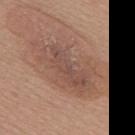Clinical impression: Recorded during total-body skin imaging; not selected for excision or biopsy. Background: A roughly 15 mm field-of-view crop from a total-body skin photograph. The total-body-photography lesion software estimated a border-irregularity index near 6/10, a within-lesion color-variation index near 4.5/10, and a peripheral color-asymmetry measure near 1.5. Approximately 9.5 mm at its widest. Captured under white-light illumination. A female patient in their mid-60s. Located on the upper back.